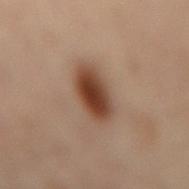biopsy status: no biopsy performed (imaged during a skin exam) | image: total-body-photography crop, ~15 mm field of view | location: the mid back | subject: female, in their mid- to late 50s.